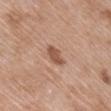{
  "biopsy_status": "not biopsied; imaged during a skin examination",
  "site": "chest",
  "image": {
    "source": "total-body photography crop",
    "field_of_view_mm": 15
  },
  "patient": {
    "sex": "female",
    "age_approx": 70
  }
}